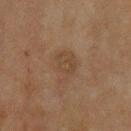Case summary:
– notes · catalogued during a skin exam; not biopsied
– image-analysis metrics · a footprint of about 7.5 mm², an eccentricity of roughly 0.6, and a shape-asymmetry score of about 0.4 (0 = symmetric); an average lesion color of about L≈35 a*≈13 b*≈25 (CIELAB) and about 5 CIELAB-L* units darker than the surrounding skin; a border-irregularity rating of about 5/10, internal color variation of about 2.5 on a 0–10 scale, and a peripheral color-asymmetry measure near 1; a nevus-likeness score of about 0/100 and lesion-presence confidence of about 100/100
– tile lighting · cross-polarized
– body site · the upper back
– subject · male, about 70 years old
– acquisition · total-body-photography crop, ~15 mm field of view